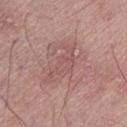No biopsy was performed on this lesion — it was imaged during a full skin examination and was not determined to be concerning. Automated tile analysis of the lesion measured a footprint of about 7.5 mm², an eccentricity of roughly 0.9, and two-axis asymmetry of about 0.5. It also reported a lesion-detection confidence of about 95/100. Longest diameter approximately 5.5 mm. On the left lower leg. A 15 mm close-up tile from a total-body photography series done for melanoma screening. The tile uses white-light illumination. A male patient, aged 53–57.